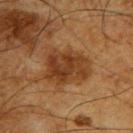follow-up = no biopsy performed (imaged during a skin exam)
imaging modality = 15 mm crop, total-body photography
illumination = cross-polarized illumination
body site = the right upper arm
patient = male, roughly 65 years of age
lesion diameter = ≈5 mm